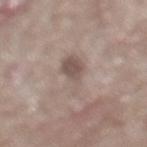- follow-up: imaged on a skin check; not biopsied
- anatomic site: the mid back
- patient: male, approximately 70 years of age
- lesion diameter: ≈6 mm
- image source: total-body-photography crop, ~15 mm field of view